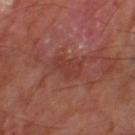{
  "biopsy_status": "not biopsied; imaged during a skin examination",
  "image": {
    "source": "total-body photography crop",
    "field_of_view_mm": 15
  },
  "automated_metrics": {
    "cielab_L": 37,
    "cielab_a": 27,
    "cielab_b": 25,
    "vs_skin_darker_L": 5.0,
    "vs_skin_contrast_norm": 5.0,
    "nevus_likeness_0_100": 0,
    "lesion_detection_confidence_0_100": 100
  },
  "site": "left thigh",
  "patient": {
    "sex": "male",
    "age_approx": 70
  }
}